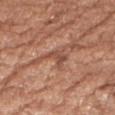Imaged during a routine full-body skin examination; the lesion was not biopsied and no histopathology is available.
A 15 mm close-up tile from a total-body photography series done for melanoma screening.
A female patient, aged 73 to 77.
This is a white-light tile.
On the right forearm.
The recorded lesion diameter is about 3 mm.